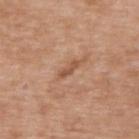  biopsy_status: not biopsied; imaged during a skin examination
  lighting: white-light
  lesion_size:
    long_diameter_mm_approx: 3.0
  automated_metrics:
    border_irregularity_0_10: 4.0
    color_variation_0_10: 0.0
    peripheral_color_asymmetry: 0.0
  patient:
    sex: male
    age_approx: 50
  site: back
  image:
    source: total-body photography crop
    field_of_view_mm: 15